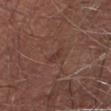Case summary:
- notes: no biopsy performed (imaged during a skin exam)
- image-analysis metrics: a shape-asymmetry score of about 0.3 (0 = symmetric); a nevus-likeness score of about 0/100
- tile lighting: white-light
- image source: 15 mm crop, total-body photography
- location: the right thigh
- patient: male, aged 58 to 62